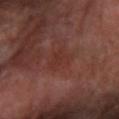Q: Was a biopsy performed?
A: catalogued during a skin exam; not biopsied
Q: What are the patient's age and sex?
A: male, aged around 70
Q: Lesion size?
A: ~3 mm (longest diameter)
Q: Automated lesion metrics?
A: a lesion area of about 5 mm², an outline eccentricity of about 0.65 (0 = round, 1 = elongated), and a shape-asymmetry score of about 0.45 (0 = symmetric); an average lesion color of about L≈31 a*≈23 b*≈24 (CIELAB), a lesion–skin lightness drop of about 4, and a normalized lesion–skin contrast near 5; internal color variation of about 1.5 on a 0–10 scale and a peripheral color-asymmetry measure near 0.5
Q: How was this image acquired?
A: total-body-photography crop, ~15 mm field of view
Q: What is the anatomic site?
A: the right forearm